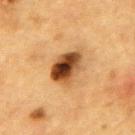Assessment: The lesion was photographed on a routine skin check and not biopsied; there is no pathology result. Clinical summary: The lesion is located on the upper back. Imaged with cross-polarized lighting. A 15 mm crop from a total-body photograph taken for skin-cancer surveillance. A male patient aged 83 or older. Longest diameter approximately 5 mm.